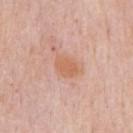- biopsy status — imaged on a skin check; not biopsied
- location — the chest
- subject — male, aged approximately 80
- image source — ~15 mm tile from a whole-body skin photo